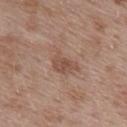Captured during whole-body skin photography for melanoma surveillance; the lesion was not biopsied.